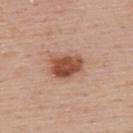Clinical impression: No biopsy was performed on this lesion — it was imaged during a full skin examination and was not determined to be concerning. Background: Located on the upper back. A male patient, roughly 55 years of age. Captured under white-light illumination. A 15 mm close-up extracted from a 3D total-body photography capture.